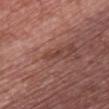Q: Was a biopsy performed?
A: imaged on a skin check; not biopsied
Q: What is the anatomic site?
A: the chest
Q: How large is the lesion?
A: ~2.5 mm (longest diameter)
Q: How was the tile lit?
A: white-light illumination
Q: Patient demographics?
A: female, about 65 years old
Q: What is the imaging modality?
A: total-body-photography crop, ~15 mm field of view